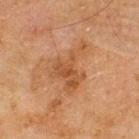<tbp_lesion>
  <biopsy_status>not biopsied; imaged during a skin examination</biopsy_status>
  <image>
    <source>total-body photography crop</source>
    <field_of_view_mm>15</field_of_view_mm>
  </image>
  <lesion_size>
    <long_diameter_mm_approx>5.5</long_diameter_mm_approx>
  </lesion_size>
  <site>upper back</site>
  <automated_metrics>
    <eccentricity>0.7</eccentricity>
    <shape_asymmetry>0.65</shape_asymmetry>
    <cielab_L>42</cielab_L>
    <cielab_a>20</cielab_a>
    <cielab_b>33</cielab_b>
    <vs_skin_contrast_norm>6.5</vs_skin_contrast_norm>
    <border_irregularity_0_10>8.0</border_irregularity_0_10>
    <peripheral_color_asymmetry>1.0</peripheral_color_asymmetry>
    <nevus_likeness_0_100>0</nevus_likeness_0_100>
    <lesion_detection_confidence_0_100>100</lesion_detection_confidence_0_100>
  </automated_metrics>
  <patient>
    <sex>male</sex>
    <age_approx>70</age_approx>
  </patient>
</tbp_lesion>